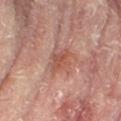Q: Was a biopsy performed?
A: catalogued during a skin exam; not biopsied
Q: Where on the body is the lesion?
A: the left leg
Q: How was this image acquired?
A: ~15 mm tile from a whole-body skin photo
Q: Patient demographics?
A: female, aged around 80
Q: Illumination type?
A: cross-polarized illumination